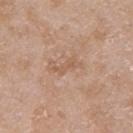<case>
  <biopsy_status>not biopsied; imaged during a skin examination</biopsy_status>
  <automated_metrics>
    <area_mm2_approx>3.5</area_mm2_approx>
    <shape_asymmetry>0.4</shape_asymmetry>
    <vs_skin_darker_L>6.0</vs_skin_darker_L>
    <peripheral_color_asymmetry>0.0</peripheral_color_asymmetry>
    <nevus_likeness_0_100>0</nevus_likeness_0_100>
    <lesion_detection_confidence_0_100>100</lesion_detection_confidence_0_100>
  </automated_metrics>
  <patient>
    <sex>female</sex>
    <age_approx>70</age_approx>
  </patient>
  <image>
    <source>total-body photography crop</source>
    <field_of_view_mm>15</field_of_view_mm>
  </image>
  <site>left upper arm</site>
  <lesion_size>
    <long_diameter_mm_approx>3.5</long_diameter_mm_approx>
  </lesion_size>
</case>